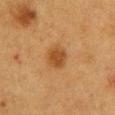Assessment: This lesion was catalogued during total-body skin photography and was not selected for biopsy. Background: The lesion is located on the chest. Imaged with cross-polarized lighting. The lesion-visualizer software estimated a border-irregularity index near 1.5/10 and a peripheral color-asymmetry measure near 1. It also reported an automated nevus-likeness rating near 95 out of 100 and lesion-presence confidence of about 100/100. This image is a 15 mm lesion crop taken from a total-body photograph. A female subject approximately 40 years of age.